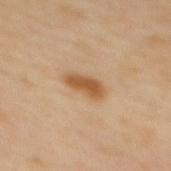Notes:
- biopsy status — no biopsy performed (imaged during a skin exam)